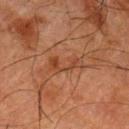* follow-up: catalogued during a skin exam; not biopsied
* anatomic site: the left thigh
* tile lighting: cross-polarized illumination
* subject: male, in their 80s
* image source: 15 mm crop, total-body photography